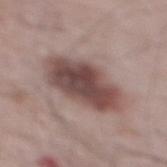This lesion was catalogued during total-body skin photography and was not selected for biopsy. Approximately 7.5 mm at its widest. From the mid back. A male subject, aged 63–67. The tile uses white-light illumination. The total-body-photography lesion software estimated a lesion area of about 24 mm². The analysis additionally found a mean CIELAB color near L≈46 a*≈18 b*≈20, roughly 15 lightness units darker than nearby skin, and a lesion-to-skin contrast of about 10.5 (normalized; higher = more distinct). The analysis additionally found a border-irregularity index near 2/10, internal color variation of about 5.5 on a 0–10 scale, and a peripheral color-asymmetry measure near 1.5. A roughly 15 mm field-of-view crop from a total-body skin photograph.